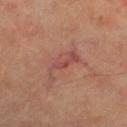Part of a total-body skin-imaging series; this lesion was reviewed on a skin check and was not flagged for biopsy.
A roughly 15 mm field-of-view crop from a total-body skin photograph.
From the leg.
The patient is a female about 60 years old.